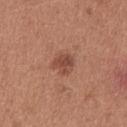workup — total-body-photography surveillance lesion; no biopsy
lighting — white-light
image source — 15 mm crop, total-body photography
subject — female, approximately 35 years of age
location — the chest